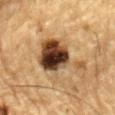* tile lighting: cross-polarized illumination
* automated metrics: a footprint of about 20 mm², a shape eccentricity near 0.8, and a shape-asymmetry score of about 0.5 (0 = symmetric); an average lesion color of about L≈37 a*≈17 b*≈29 (CIELAB); a border-irregularity rating of about 6/10, internal color variation of about 10 on a 0–10 scale, and a peripheral color-asymmetry measure near 3.5; an automated nevus-likeness rating near 90 out of 100 and a lesion-detection confidence of about 100/100
* lesion diameter: ~7.5 mm (longest diameter)
* subject: male, roughly 85 years of age or older
* body site: the mid back
* acquisition: total-body-photography crop, ~15 mm field of view